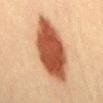Assessment:
Imaged during a routine full-body skin examination; the lesion was not biopsied and no histopathology is available.
Context:
A male subject in their 40s. The lesion is on the abdomen. A 15 mm crop from a total-body photograph taken for skin-cancer surveillance.